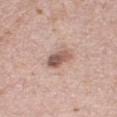  biopsy_status: not biopsied; imaged during a skin examination
  lighting: white-light
  site: right thigh
  image:
    source: total-body photography crop
    field_of_view_mm: 15
  automated_metrics:
    area_mm2_approx: 5.5
    eccentricity: 0.85
    shape_asymmetry: 0.15
    border_irregularity_0_10: 1.5
    color_variation_0_10: 6.0
    peripheral_color_asymmetry: 2.0
  lesion_size:
    long_diameter_mm_approx: 3.5
  patient:
    sex: female
    age_approx: 40